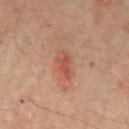Clinical summary: An algorithmic analysis of the crop reported a border-irregularity index near 3/10 and internal color variation of about 2 on a 0–10 scale. Located on the back. The tile uses cross-polarized illumination. A male subject, roughly 65 years of age. A 15 mm close-up extracted from a 3D total-body photography capture. Longest diameter approximately 4 mm.